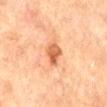follow-up: imaged on a skin check; not biopsied | lesion size: ~3.5 mm (longest diameter) | patient: male, in their 70s | location: the mid back | TBP lesion metrics: a lesion area of about 5.5 mm², an eccentricity of roughly 0.85, and a symmetry-axis asymmetry near 0.25; a mean CIELAB color near L≈52 a*≈24 b*≈35, a lesion–skin lightness drop of about 12, and a normalized lesion–skin contrast near 8.5 | image: 15 mm crop, total-body photography.